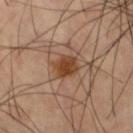workup = no biopsy performed (imaged during a skin exam)
illumination = cross-polarized
location = the right thigh
lesion diameter = ≈3 mm
subject = male, about 60 years old
acquisition = 15 mm crop, total-body photography